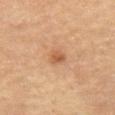Imaged during a routine full-body skin examination; the lesion was not biopsied and no histopathology is available. The subject is a female in their mid- to late 60s. Located on the left thigh. Captured under cross-polarized illumination. A region of skin cropped from a whole-body photographic capture, roughly 15 mm wide. Approximately 2 mm at its widest.